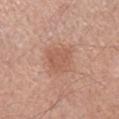{"biopsy_status": "not biopsied; imaged during a skin examination", "lesion_size": {"long_diameter_mm_approx": 4.0}, "image": {"source": "total-body photography crop", "field_of_view_mm": 15}, "site": "left forearm", "lighting": "white-light", "automated_metrics": {"vs_skin_darker_L": 7.0, "vs_skin_contrast_norm": 5.0, "color_variation_0_10": 2.0, "peripheral_color_asymmetry": 0.5}, "patient": {"sex": "male", "age_approx": 60}}